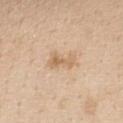This lesion was catalogued during total-body skin photography and was not selected for biopsy. The lesion is on the chest. Measured at roughly 3.5 mm in maximum diameter. This is a white-light tile. Automated tile analysis of the lesion measured an area of roughly 5.5 mm², an eccentricity of roughly 0.85, and a shape-asymmetry score of about 0.4 (0 = symmetric). And it measured an average lesion color of about L≈65 a*≈17 b*≈35 (CIELAB) and a lesion–skin lightness drop of about 9. The software also gave a color-variation rating of about 3.5/10 and a peripheral color-asymmetry measure near 1. And it measured a classifier nevus-likeness of about 5/100 and lesion-presence confidence of about 100/100. A female subject, aged 53 to 57. A 15 mm crop from a total-body photograph taken for skin-cancer surveillance.